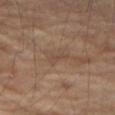Q: Was this lesion biopsied?
A: no biopsy performed (imaged during a skin exam)
Q: How was the tile lit?
A: cross-polarized
Q: What is the anatomic site?
A: the right thigh
Q: What is the imaging modality?
A: total-body-photography crop, ~15 mm field of view
Q: How large is the lesion?
A: ~2.5 mm (longest diameter)
Q: Who is the patient?
A: male, about 70 years old